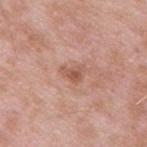Clinical summary:
Measured at roughly 2.5 mm in maximum diameter. A 15 mm crop from a total-body photograph taken for skin-cancer surveillance. Captured under white-light illumination. The lesion is located on the arm. A male patient about 55 years old.